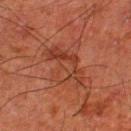Background: This image is a 15 mm lesion crop taken from a total-body photograph. An algorithmic analysis of the crop reported a mean CIELAB color near L≈32 a*≈23 b*≈27 and roughly 5 lightness units darker than nearby skin. It also reported a classifier nevus-likeness of about 0/100 and a detector confidence of about 100 out of 100 that the crop contains a lesion. Measured at roughly 5.5 mm in maximum diameter. From the left lower leg. The subject is a male aged 78–82.